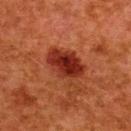notes: no biopsy performed (imaged during a skin exam) | lesion diameter: ~5 mm (longest diameter) | site: the upper back | image: 15 mm crop, total-body photography | TBP lesion metrics: a lesion area of about 13 mm², an outline eccentricity of about 0.75 (0 = round, 1 = elongated), and a symmetry-axis asymmetry near 0.25; a lesion color around L≈27 a*≈28 b*≈29 in CIELAB and roughly 11 lightness units darker than nearby skin | illumination: cross-polarized illumination | subject: female, about 50 years old.